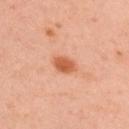The lesion was tiled from a total-body skin photograph and was not biopsied.
A male patient, aged around 45.
About 3 mm across.
The total-body-photography lesion software estimated an average lesion color of about L≈58 a*≈29 b*≈37 (CIELAB) and about 12 CIELAB-L* units darker than the surrounding skin. The analysis additionally found lesion-presence confidence of about 100/100.
Captured under cross-polarized illumination.
From the upper back.
Cropped from a whole-body photographic skin survey; the tile spans about 15 mm.